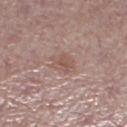The lesion was tiled from a total-body skin photograph and was not biopsied.
The recorded lesion diameter is about 3 mm.
Automated tile analysis of the lesion measured an area of roughly 6 mm², an outline eccentricity of about 0.6 (0 = round, 1 = elongated), and a symmetry-axis asymmetry near 0.25. It also reported a border-irregularity index near 3/10, internal color variation of about 2 on a 0–10 scale, and peripheral color asymmetry of about 0.5.
A female subject about 65 years old.
The lesion is located on the left lower leg.
A 15 mm crop from a total-body photograph taken for skin-cancer surveillance.
The tile uses white-light illumination.